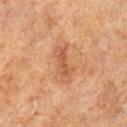{
  "biopsy_status": "not biopsied; imaged during a skin examination"
}